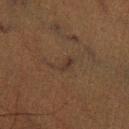Case summary:
– biopsy status — catalogued during a skin exam; not biopsied
– imaging modality — 15 mm crop, total-body photography
– anatomic site — the left lower leg
– illumination — cross-polarized illumination
– patient — male, roughly 50 years of age
– automated metrics — an average lesion color of about L≈26 a*≈12 b*≈20 (CIELAB)